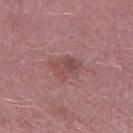Clinical impression: Recorded during total-body skin imaging; not selected for excision or biopsy. Image and clinical context: The lesion-visualizer software estimated an area of roughly 7 mm² and two-axis asymmetry of about 0.25. The software also gave a lesion color around L≈48 a*≈23 b*≈21 in CIELAB and a normalized border contrast of about 6. And it measured lesion-presence confidence of about 100/100. A 15 mm close-up extracted from a 3D total-body photography capture. Approximately 3.5 mm at its widest. A male patient aged 53–57. The lesion is on the leg.